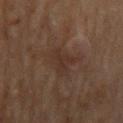Assessment: This lesion was catalogued during total-body skin photography and was not selected for biopsy. Clinical summary: The total-body-photography lesion software estimated a lesion color around L≈23 a*≈12 b*≈18 in CIELAB and roughly 4 lightness units darker than nearby skin. And it measured a border-irregularity index near 3.5/10, a within-lesion color-variation index near 2/10, and a peripheral color-asymmetry measure near 0.5. A 15 mm close-up extracted from a 3D total-body photography capture. A male patient aged 68–72. The tile uses cross-polarized illumination. Measured at roughly 4 mm in maximum diameter. On the mid back.